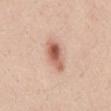follow-up: catalogued during a skin exam; not biopsied
diameter: about 4.5 mm
TBP lesion metrics: a lesion area of about 7.5 mm², an outline eccentricity of about 0.9 (0 = round, 1 = elongated), and a symmetry-axis asymmetry near 0.2
body site: the back
patient: male, aged approximately 25
image: total-body-photography crop, ~15 mm field of view